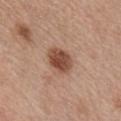<tbp_lesion>
<biopsy_status>not biopsied; imaged during a skin examination</biopsy_status>
<site>chest</site>
<patient>
  <sex>male</sex>
  <age_approx>40</age_approx>
</patient>
<lesion_size>
  <long_diameter_mm_approx>3.5</long_diameter_mm_approx>
</lesion_size>
<lighting>white-light</lighting>
<image>
  <source>total-body photography crop</source>
  <field_of_view_mm>15</field_of_view_mm>
</image>
</tbp_lesion>